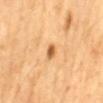biopsy_status: not biopsied; imaged during a skin examination
lesion_size:
  long_diameter_mm_approx: 2.0
patient:
  sex: male
  age_approx: 65
lighting: cross-polarized
image:
  source: total-body photography crop
  field_of_view_mm: 15
site: mid back